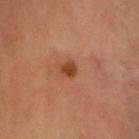Impression:
The lesion was photographed on a routine skin check and not biopsied; there is no pathology result.
Clinical summary:
Imaged with cross-polarized lighting. A 15 mm crop from a total-body photograph taken for skin-cancer surveillance. From the head or neck. A male patient, aged 43 to 47. Measured at roughly 2 mm in maximum diameter. Automated image analysis of the tile measured an average lesion color of about L≈44 a*≈27 b*≈36 (CIELAB), about 11 CIELAB-L* units darker than the surrounding skin, and a normalized lesion–skin contrast near 8.5.